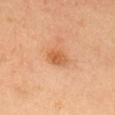follow-up — imaged on a skin check; not biopsied | tile lighting — cross-polarized | subject — female, aged 33–37 | imaging modality — 15 mm crop, total-body photography | anatomic site — the head or neck.